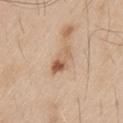Q: Was this lesion biopsied?
A: catalogued during a skin exam; not biopsied
Q: Lesion size?
A: ≈3.5 mm
Q: What kind of image is this?
A: ~15 mm crop, total-body skin-cancer survey
Q: How was the tile lit?
A: white-light illumination
Q: Where on the body is the lesion?
A: the left thigh
Q: Who is the patient?
A: male, approximately 60 years of age